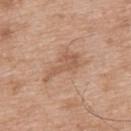This lesion was catalogued during total-body skin photography and was not selected for biopsy.